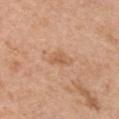Imaged during a routine full-body skin examination; the lesion was not biopsied and no histopathology is available. This image is a 15 mm lesion crop taken from a total-body photograph. The subject is a male roughly 70 years of age. The lesion is on the arm. This is a white-light tile.